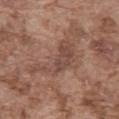Case summary:
• biopsy status · catalogued during a skin exam; not biopsied
• subject · male, aged 73 to 77
• body site · the abdomen
• image · total-body-photography crop, ~15 mm field of view
• tile lighting · white-light illumination